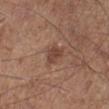workup=total-body-photography surveillance lesion; no biopsy | anatomic site=the left lower leg | patient=male, aged around 60 | illumination=white-light | lesion size=≈3 mm | acquisition=15 mm crop, total-body photography | TBP lesion metrics=a symmetry-axis asymmetry near 0.35; an automated nevus-likeness rating near 30 out of 100.